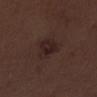Clinical impression:
The lesion was photographed on a routine skin check and not biopsied; there is no pathology result.
Background:
A roughly 15 mm field-of-view crop from a total-body skin photograph. The subject is a male in their 70s. This is a white-light tile. The lesion is on the right lower leg.